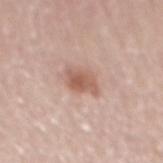The lesion was photographed on a routine skin check and not biopsied; there is no pathology result. Imaged with white-light lighting. Cropped from a total-body skin-imaging series; the visible field is about 15 mm. On the mid back. Longest diameter approximately 3 mm. A male patient about 60 years old.